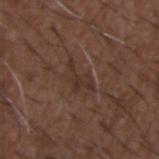<case>
<biopsy_status>not biopsied; imaged during a skin examination</biopsy_status>
<site>upper back</site>
<image>
  <source>total-body photography crop</source>
  <field_of_view_mm>15</field_of_view_mm>
</image>
<patient>
  <sex>male</sex>
  <age_approx>50</age_approx>
</patient>
</case>